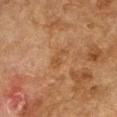Findings:
• patient: female, aged 58 to 62
• lesion size: ≈2.5 mm
• lighting: cross-polarized illumination
• acquisition: 15 mm crop, total-body photography
• body site: the right upper arm